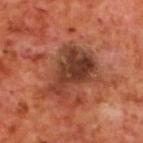Impression: No biopsy was performed on this lesion — it was imaged during a full skin examination and was not determined to be concerning. Background: The lesion is located on the upper back. A 15 mm close-up extracted from a 3D total-body photography capture. Automated image analysis of the tile measured internal color variation of about 6.5 on a 0–10 scale. Approximately 6.5 mm at its widest. A male patient in their 70s.